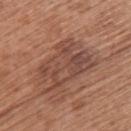Impression:
The lesion was tiled from a total-body skin photograph and was not biopsied.
Image and clinical context:
A 15 mm close-up extracted from a 3D total-body photography capture. A female patient, aged 63–67. Located on the upper back.